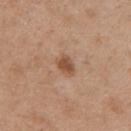lighting: white-light
patient:
  sex: female
  age_approx: 40
site: mid back
lesion_size:
  long_diameter_mm_approx: 3.0
automated_metrics:
  border_irregularity_0_10: 2.5
  peripheral_color_asymmetry: 0.5
image:
  source: total-body photography crop
  field_of_view_mm: 15